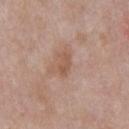workup = total-body-photography surveillance lesion; no biopsy.